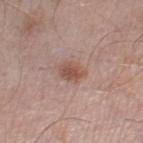The tile uses white-light illumination. The recorded lesion diameter is about 3 mm. A male patient aged approximately 55. The lesion-visualizer software estimated an outline eccentricity of about 0.75 (0 = round, 1 = elongated) and two-axis asymmetry of about 0.2. And it measured a border-irregularity rating of about 2/10 and peripheral color asymmetry of about 0.5. It also reported an automated nevus-likeness rating near 85 out of 100 and lesion-presence confidence of about 100/100. A region of skin cropped from a whole-body photographic capture, roughly 15 mm wide. On the leg.